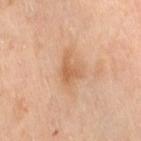Measured at roughly 4.5 mm in maximum diameter. A lesion tile, about 15 mm wide, cut from a 3D total-body photograph. Automated image analysis of the tile measured a footprint of about 8.5 mm² and a symmetry-axis asymmetry near 0.35. The analysis additionally found a mean CIELAB color near L≈64 a*≈21 b*≈37, about 8 CIELAB-L* units darker than the surrounding skin, and a normalized border contrast of about 6. It also reported radial color variation of about 1.5. The tile uses cross-polarized illumination. A female subject aged approximately 55. From the leg.